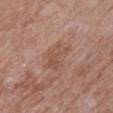Q: Is there a histopathology result?
A: no biopsy performed (imaged during a skin exam)